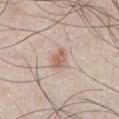workup: imaged on a skin check; not biopsied | image source: ~15 mm crop, total-body skin-cancer survey | patient: male, aged 43–47 | anatomic site: the abdomen.